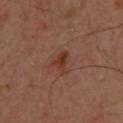<tbp_lesion>
<biopsy_status>not biopsied; imaged during a skin examination</biopsy_status>
<image>
  <source>total-body photography crop</source>
  <field_of_view_mm>15</field_of_view_mm>
</image>
<patient>
  <sex>male</sex>
  <age_approx>55</age_approx>
</patient>
<site>upper back</site>
</tbp_lesion>